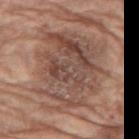This lesion was catalogued during total-body skin photography and was not selected for biopsy. A 15 mm crop from a total-body photograph taken for skin-cancer surveillance. Automated tile analysis of the lesion measured an area of roughly 55 mm², an eccentricity of roughly 0.65, and a shape-asymmetry score of about 0.25 (0 = symmetric). The analysis additionally found a nevus-likeness score of about 20/100 and lesion-presence confidence of about 65/100. Imaged with white-light lighting. The lesion is on the left thigh. A female patient, in their mid- to late 70s.